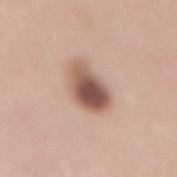Clinical impression:
The lesion was photographed on a routine skin check and not biopsied; there is no pathology result.
Acquisition and patient details:
A 15 mm close-up tile from a total-body photography series done for melanoma screening. The tile uses white-light illumination. The patient is a female about 50 years old. The lesion is on the mid back. Measured at roughly 5.5 mm in maximum diameter. Automated image analysis of the tile measured a lesion area of about 13 mm², an eccentricity of roughly 0.85, and two-axis asymmetry of about 0.2. It also reported an average lesion color of about L≈55 a*≈19 b*≈26 (CIELAB), about 16 CIELAB-L* units darker than the surrounding skin, and a normalized border contrast of about 10.